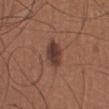notes — no biopsy performed (imaged during a skin exam)
imaging modality — ~15 mm tile from a whole-body skin photo
lighting — white-light
location — the right lower leg
patient — male, aged 63 to 67
lesion size — about 3.5 mm
TBP lesion metrics — two-axis asymmetry of about 0.1; a lesion color around L≈38 a*≈20 b*≈24 in CIELAB and a normalized border contrast of about 9.5; a border-irregularity index near 1/10; lesion-presence confidence of about 100/100